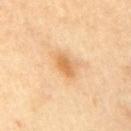Assessment:
The lesion was photographed on a routine skin check and not biopsied; there is no pathology result.
Acquisition and patient details:
The patient is a male in their mid- to late 60s. The tile uses cross-polarized illumination. The lesion is on the right upper arm. A 15 mm crop from a total-body photograph taken for skin-cancer surveillance. Automated tile analysis of the lesion measured an eccentricity of roughly 0.85 and a shape-asymmetry score of about 0.2 (0 = symmetric). It also reported an average lesion color of about L≈65 a*≈21 b*≈43 (CIELAB). It also reported a classifier nevus-likeness of about 55/100 and a detector confidence of about 100 out of 100 that the crop contains a lesion. Measured at roughly 3 mm in maximum diameter.